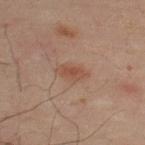Assessment:
This lesion was catalogued during total-body skin photography and was not selected for biopsy.
Clinical summary:
The tile uses cross-polarized illumination. Located on the upper back. The lesion-visualizer software estimated an area of roughly 6 mm² and a shape-asymmetry score of about 0.3 (0 = symmetric). And it measured a border-irregularity rating of about 3/10, a color-variation rating of about 2/10, and a peripheral color-asymmetry measure near 0.5. And it measured a lesion-detection confidence of about 100/100. A male subject, about 50 years old. The recorded lesion diameter is about 3.5 mm. Cropped from a total-body skin-imaging series; the visible field is about 15 mm.